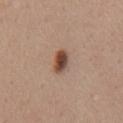{
  "biopsy_status": "not biopsied; imaged during a skin examination",
  "site": "front of the torso",
  "lighting": "white-light",
  "image": {
    "source": "total-body photography crop",
    "field_of_view_mm": 15
  },
  "lesion_size": {
    "long_diameter_mm_approx": 3.0
  },
  "patient": {
    "sex": "female",
    "age_approx": 55
  }
}